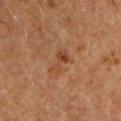Case summary:
• workup: no biopsy performed (imaged during a skin exam)
• lesion size: ≈3 mm
• subject: male, about 60 years old
• imaging modality: ~15 mm crop, total-body skin-cancer survey
• site: the chest